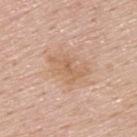biopsy status = imaged on a skin check; not biopsied | image source = ~15 mm crop, total-body skin-cancer survey | patient = male, in their mid-50s | anatomic site = the back | size = ~5 mm (longest diameter) | image-analysis metrics = a footprint of about 9.5 mm² and a shape eccentricity near 0.8; a nevus-likeness score of about 0/100 and lesion-presence confidence of about 100/100.